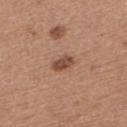Impression: The lesion was photographed on a routine skin check and not biopsied; there is no pathology result. Acquisition and patient details: Longest diameter approximately 2.5 mm. A female subject, aged approximately 35. Located on the upper back. The tile uses white-light illumination. This image is a 15 mm lesion crop taken from a total-body photograph.